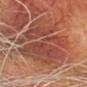Recorded during total-body skin imaging; not selected for excision or biopsy.
The recorded lesion diameter is about 11.5 mm.
A 15 mm close-up tile from a total-body photography series done for melanoma screening.
The lesion is on the head or neck.
Imaged with cross-polarized lighting.
The subject is a male about 80 years old.